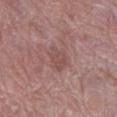The lesion was tiled from a total-body skin photograph and was not biopsied. A close-up tile cropped from a whole-body skin photograph, about 15 mm across. A female patient, approximately 70 years of age. The lesion's longest dimension is about 3 mm. The lesion is located on the left lower leg. Imaged with white-light lighting.